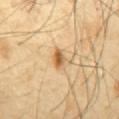notes: total-body-photography surveillance lesion; no biopsy
subject: male, in their mid- to late 60s
size: ≈2.5 mm
acquisition: total-body-photography crop, ~15 mm field of view
body site: the abdomen
illumination: cross-polarized